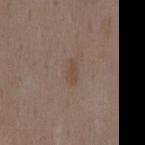This lesion was catalogued during total-body skin photography and was not selected for biopsy.
Cropped from a total-body skin-imaging series; the visible field is about 15 mm.
A female patient aged 33 to 37.
This is a white-light tile.
Located on the mid back.
About 2.5 mm across.